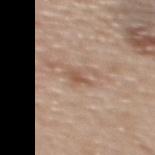Clinical impression: Imaged during a routine full-body skin examination; the lesion was not biopsied and no histopathology is available. Context: The lesion's longest dimension is about 2.5 mm. A 15 mm close-up extracted from a 3D total-body photography capture. A male subject aged 68 to 72. On the upper back. This is a white-light tile.